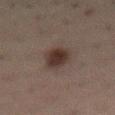workup: imaged on a skin check; not biopsied
patient: male, aged around 50
anatomic site: the abdomen
image: total-body-photography crop, ~15 mm field of view
diameter: about 4 mm
illumination: cross-polarized illumination